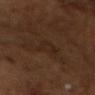  biopsy_status: not biopsied; imaged during a skin examination
  site: head or neck
  image:
    source: total-body photography crop
    field_of_view_mm: 15
  patient:
    sex: male
    age_approx: 55